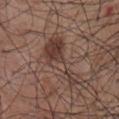This lesion was catalogued during total-body skin photography and was not selected for biopsy. A close-up tile cropped from a whole-body skin photograph, about 15 mm across. A male subject, aged 53–57. On the front of the torso.